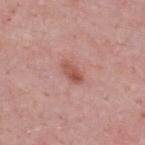Impression: This lesion was catalogued during total-body skin photography and was not selected for biopsy.